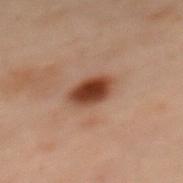Impression: No biopsy was performed on this lesion — it was imaged during a full skin examination and was not determined to be concerning. Image and clinical context: About 3.5 mm across. A 15 mm close-up tile from a total-body photography series done for melanoma screening. The patient is a female roughly 55 years of age. The lesion is located on the upper back. This is a cross-polarized tile.